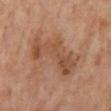No biopsy was performed on this lesion — it was imaged during a full skin examination and was not determined to be concerning. From the mid back. The patient is a male aged around 65. This is a cross-polarized tile. The lesion-visualizer software estimated a lesion area of about 17 mm², an outline eccentricity of about 0.95 (0 = round, 1 = elongated), and two-axis asymmetry of about 0.5. Cropped from a total-body skin-imaging series; the visible field is about 15 mm. About 8 mm across.